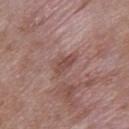follow-up=imaged on a skin check; not biopsied | subject=male, aged 48–52 | image=15 mm crop, total-body photography | site=the upper back.